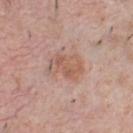{"biopsy_status": "not biopsied; imaged during a skin examination", "patient": {"sex": "male", "age_approx": 40}, "site": "chest", "image": {"source": "total-body photography crop", "field_of_view_mm": 15}, "lighting": "white-light", "lesion_size": {"long_diameter_mm_approx": 4.0}, "automated_metrics": {"border_irregularity_0_10": 3.0, "color_variation_0_10": 4.5, "peripheral_color_asymmetry": 1.5, "nevus_likeness_0_100": 10}}